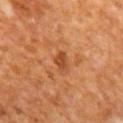Q: Was this lesion biopsied?
A: no biopsy performed (imaged during a skin exam)
Q: Lesion location?
A: the mid back
Q: What kind of image is this?
A: ~15 mm tile from a whole-body skin photo
Q: Patient demographics?
A: male, about 65 years old